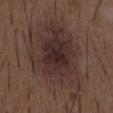follow-up — total-body-photography surveillance lesion; no biopsy
acquisition — ~15 mm tile from a whole-body skin photo
subject — male, in their 50s
tile lighting — white-light illumination
site — the chest